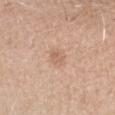Assessment: Imaged during a routine full-body skin examination; the lesion was not biopsied and no histopathology is available. Context: From the head or neck. About 2.5 mm across. The lesion-visualizer software estimated a lesion color around L≈63 a*≈19 b*≈31 in CIELAB, a lesion–skin lightness drop of about 7, and a normalized lesion–skin contrast near 5. It also reported a border-irregularity index near 1.5/10, a within-lesion color-variation index near 2/10, and a peripheral color-asymmetry measure near 0.5. And it measured an automated nevus-likeness rating near 5 out of 100 and lesion-presence confidence of about 100/100. Cropped from a total-body skin-imaging series; the visible field is about 15 mm. A male patient in their 30s.